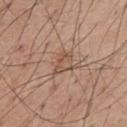Recorded during total-body skin imaging; not selected for excision or biopsy. Cropped from a total-body skin-imaging series; the visible field is about 15 mm. From the mid back. An algorithmic analysis of the crop reported a footprint of about 5.5 mm², an outline eccentricity of about 0.7 (0 = round, 1 = elongated), and a shape-asymmetry score of about 0.25 (0 = symmetric). It also reported a mean CIELAB color near L≈53 a*≈18 b*≈28, roughly 7 lightness units darker than nearby skin, and a lesion-to-skin contrast of about 5.5 (normalized; higher = more distinct). This is a white-light tile. A male subject aged around 55.